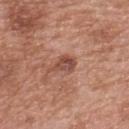Impression:
Part of a total-body skin-imaging series; this lesion was reviewed on a skin check and was not flagged for biopsy.
Clinical summary:
Cropped from a whole-body photographic skin survey; the tile spans about 15 mm. The lesion is located on the upper back. A female subject, aged approximately 60.